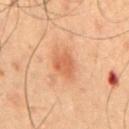Assessment:
No biopsy was performed on this lesion — it was imaged during a full skin examination and was not determined to be concerning.
Clinical summary:
A 15 mm crop from a total-body photograph taken for skin-cancer surveillance. The lesion is on the abdomen. The subject is a male aged around 50.